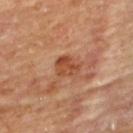Part of a total-body skin-imaging series; this lesion was reviewed on a skin check and was not flagged for biopsy. The lesion-visualizer software estimated an average lesion color of about L≈47 a*≈26 b*≈34 (CIELAB), a lesion–skin lightness drop of about 9, and a normalized lesion–skin contrast near 7.5. The software also gave a border-irregularity index near 2/10 and a peripheral color-asymmetry measure near 1. The software also gave a nevus-likeness score of about 0/100 and a detector confidence of about 100 out of 100 that the crop contains a lesion. Longest diameter approximately 3 mm. Located on the upper back. The subject is a male aged approximately 85 or older. This image is a 15 mm lesion crop taken from a total-body photograph.